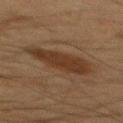Assessment:
The lesion was tiled from a total-body skin photograph and was not biopsied.
Clinical summary:
Imaged with cross-polarized lighting. Approximately 6.5 mm at its widest. Cropped from a whole-body photographic skin survey; the tile spans about 15 mm. The subject is a male aged approximately 65. The lesion is on the mid back. The total-body-photography lesion software estimated an average lesion color of about L≈29 a*≈15 b*≈25 (CIELAB), about 8 CIELAB-L* units darker than the surrounding skin, and a lesion-to-skin contrast of about 8 (normalized; higher = more distinct). It also reported a color-variation rating of about 2/10 and a peripheral color-asymmetry measure near 0.5. And it measured a classifier nevus-likeness of about 35/100 and a lesion-detection confidence of about 100/100.